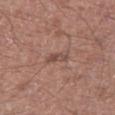About 2.5 mm across. Located on the left thigh. Cropped from a total-body skin-imaging series; the visible field is about 15 mm. This is a white-light tile. An algorithmic analysis of the crop reported an eccentricity of roughly 0.9 and two-axis asymmetry of about 0.4. And it measured a border-irregularity rating of about 4.5/10, a within-lesion color-variation index near 2/10, and peripheral color asymmetry of about 0.5. The analysis additionally found a classifier nevus-likeness of about 0/100 and a detector confidence of about 100 out of 100 that the crop contains a lesion. A male subject about 55 years old.